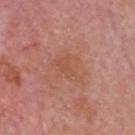Image and clinical context: The lesion is on the head or neck. Automated tile analysis of the lesion measured a detector confidence of about 100 out of 100 that the crop contains a lesion. A male patient aged 73 to 77. Captured under white-light illumination. Cropped from a whole-body photographic skin survey; the tile spans about 15 mm. The recorded lesion diameter is about 4.5 mm.